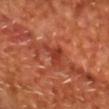- follow-up: imaged on a skin check; not biopsied
- acquisition: 15 mm crop, total-body photography
- diameter: about 3.5 mm
- patient: male, aged 63 to 67
- tile lighting: cross-polarized illumination